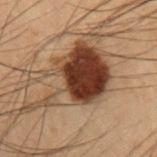Q: Was a biopsy performed?
A: catalogued during a skin exam; not biopsied